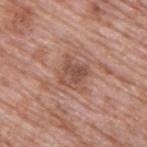patient:
  sex: male
  age_approx: 70
site: upper back
lesion_size:
  long_diameter_mm_approx: 3.0
image:
  source: total-body photography crop
  field_of_view_mm: 15